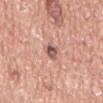The lesion was tiled from a total-body skin photograph and was not biopsied. On the abdomen. The subject is a male in their mid-70s. A roughly 15 mm field-of-view crop from a total-body skin photograph.